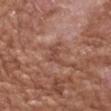The lesion was tiled from a total-body skin photograph and was not biopsied. On the left forearm. A male patient, about 65 years old. A close-up tile cropped from a whole-body skin photograph, about 15 mm across. The tile uses white-light illumination. About 3 mm across. The total-body-photography lesion software estimated a border-irregularity rating of about 6/10. The analysis additionally found a nevus-likeness score of about 0/100 and lesion-presence confidence of about 75/100.